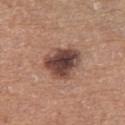- acquisition: ~15 mm crop, total-body skin-cancer survey
- site: the right thigh
- patient: female, in their mid- to late 50s